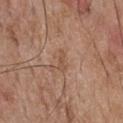{"biopsy_status": "not biopsied; imaged during a skin examination", "patient": {"sex": "male", "age_approx": 55}, "lesion_size": {"long_diameter_mm_approx": 2.5}, "lighting": "white-light", "image": {"source": "total-body photography crop", "field_of_view_mm": 15}, "site": "chest", "automated_metrics": {"cielab_L": 51, "cielab_a": 19, "cielab_b": 29, "vs_skin_contrast_norm": 5.5, "peripheral_color_asymmetry": 0.0}}